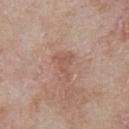The lesion was tiled from a total-body skin photograph and was not biopsied.
Located on the chest.
Automated tile analysis of the lesion measured a shape-asymmetry score of about 0.6 (0 = symmetric). It also reported roughly 7 lightness units darker than nearby skin and a normalized lesion–skin contrast near 5. And it measured a border-irregularity index near 6/10, internal color variation of about 2.5 on a 0–10 scale, and radial color variation of about 0.5. The software also gave a nevus-likeness score of about 0/100.
Longest diameter approximately 3.5 mm.
This is a white-light tile.
Cropped from a total-body skin-imaging series; the visible field is about 15 mm.
The patient is a male approximately 65 years of age.